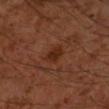Assessment:
Imaged during a routine full-body skin examination; the lesion was not biopsied and no histopathology is available.
Clinical summary:
A lesion tile, about 15 mm wide, cut from a 3D total-body photograph. The subject is a male aged 58 to 62. The lesion is on the arm. Approximately 3 mm at its widest.